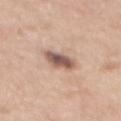| key | value |
|---|---|
| biopsy status | total-body-photography surveillance lesion; no biopsy |
| lesion size | about 4.5 mm |
| subject | female, aged around 40 |
| acquisition | 15 mm crop, total-body photography |
| body site | the mid back |